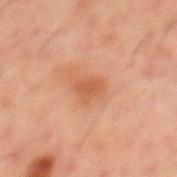No biopsy was performed on this lesion — it was imaged during a full skin examination and was not determined to be concerning.
The total-body-photography lesion software estimated a footprint of about 4.5 mm², an outline eccentricity of about 0.6 (0 = round, 1 = elongated), and a shape-asymmetry score of about 0.35 (0 = symmetric). The software also gave a border-irregularity index near 3/10, internal color variation of about 1.5 on a 0–10 scale, and radial color variation of about 0.5. And it measured lesion-presence confidence of about 100/100.
A male patient, about 50 years old.
Longest diameter approximately 2.5 mm.
From the back.
A roughly 15 mm field-of-view crop from a total-body skin photograph.
Imaged with cross-polarized lighting.